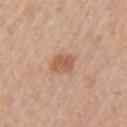Q: Was a biopsy performed?
A: catalogued during a skin exam; not biopsied
Q: Where on the body is the lesion?
A: the left upper arm
Q: What are the patient's age and sex?
A: female, aged 48 to 52
Q: How large is the lesion?
A: ≈3.5 mm
Q: How was the tile lit?
A: white-light
Q: What is the imaging modality?
A: ~15 mm crop, total-body skin-cancer survey